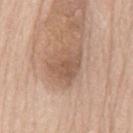Imaged with white-light lighting. On the mid back. A lesion tile, about 15 mm wide, cut from a 3D total-body photograph. Measured at roughly 4.5 mm in maximum diameter. A female subject approximately 75 years of age.